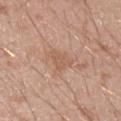Assessment: The lesion was photographed on a routine skin check and not biopsied; there is no pathology result. Context: The recorded lesion diameter is about 3 mm. A roughly 15 mm field-of-view crop from a total-body skin photograph. A male patient, approximately 20 years of age. The tile uses white-light illumination. The lesion is located on the right upper arm.